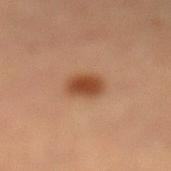workup: total-body-photography surveillance lesion; no biopsy | TBP lesion metrics: a lesion area of about 7 mm², an outline eccentricity of about 0.6 (0 = round, 1 = elongated), and a symmetry-axis asymmetry near 0.15; a mean CIELAB color near L≈41 a*≈21 b*≈31, a lesion–skin lightness drop of about 11, and a normalized lesion–skin contrast near 9.5 | acquisition: total-body-photography crop, ~15 mm field of view | subject: female, aged around 60 | illumination: cross-polarized illumination | location: the left lower leg.